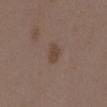<tbp_lesion>
<biopsy_status>not biopsied; imaged during a skin examination</biopsy_status>
<site>front of the torso</site>
<image>
  <source>total-body photography crop</source>
  <field_of_view_mm>15</field_of_view_mm>
</image>
<automated_metrics>
  <cielab_L>43</cielab_L>
  <cielab_a>15</cielab_a>
  <cielab_b>25</cielab_b>
  <vs_skin_darker_L>7.0</vs_skin_darker_L>
  <border_irregularity_0_10>2.5</border_irregularity_0_10>
  <peripheral_color_asymmetry>0.5</peripheral_color_asymmetry>
</automated_metrics>
<lighting>white-light</lighting>
<lesion_size>
  <long_diameter_mm_approx>2.5</long_diameter_mm_approx>
</lesion_size>
<patient>
  <sex>female</sex>
  <age_approx>35</age_approx>
</patient>
</tbp_lesion>